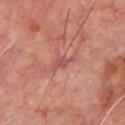The lesion was tiled from a total-body skin photograph and was not biopsied.
A male subject, aged 63 to 67.
Cropped from a whole-body photographic skin survey; the tile spans about 15 mm.
The lesion is on the front of the torso.